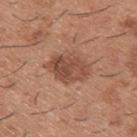Imaged during a routine full-body skin examination; the lesion was not biopsied and no histopathology is available. Longest diameter approximately 4.5 mm. The lesion is located on the upper back. A 15 mm crop from a total-body photograph taken for skin-cancer surveillance. A male patient, aged around 40. The tile uses white-light illumination.